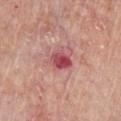follow-up: total-body-photography surveillance lesion; no biopsy | patient: male, aged around 65 | anatomic site: the chest | image source: ~15 mm tile from a whole-body skin photo.